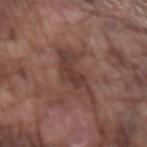Assessment: Recorded during total-body skin imaging; not selected for excision or biopsy. Image and clinical context: A male subject in their mid- to late 70s. Located on the left forearm. The lesion-visualizer software estimated an average lesion color of about L≈38 a*≈18 b*≈22 (CIELAB), a lesion–skin lightness drop of about 8, and a normalized border contrast of about 7. And it measured a border-irregularity rating of about 8/10, a color-variation rating of about 3/10, and radial color variation of about 0.5. A 15 mm close-up extracted from a 3D total-body photography capture.